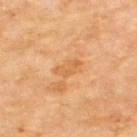Q: Was a biopsy performed?
A: total-body-photography surveillance lesion; no biopsy
Q: What lighting was used for the tile?
A: cross-polarized
Q: Patient demographics?
A: male, roughly 70 years of age
Q: Automated lesion metrics?
A: an outline eccentricity of about 0.85 (0 = round, 1 = elongated) and a shape-asymmetry score of about 0.35 (0 = symmetric); an average lesion color of about L≈62 a*≈24 b*≈44 (CIELAB) and a normalized lesion–skin contrast near 5.5; border irregularity of about 4 on a 0–10 scale and peripheral color asymmetry of about 0
Q: Lesion location?
A: the upper back
Q: What is the lesion's diameter?
A: ~3 mm (longest diameter)
Q: How was this image acquired?
A: 15 mm crop, total-body photography